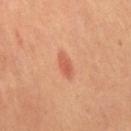Q: Was a biopsy performed?
A: total-body-photography surveillance lesion; no biopsy
Q: What is the imaging modality?
A: 15 mm crop, total-body photography
Q: What is the anatomic site?
A: the left leg
Q: Who is the patient?
A: female, in their mid- to late 60s